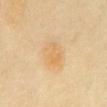The lesion was tiled from a total-body skin photograph and was not biopsied. Approximately 4 mm at its widest. Located on the chest. This is a cross-polarized tile. A roughly 15 mm field-of-view crop from a total-body skin photograph. A female patient, aged 58–62. The lesion-visualizer software estimated an automated nevus-likeness rating near 20 out of 100 and lesion-presence confidence of about 100/100.